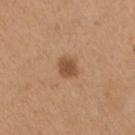The lesion was tiled from a total-body skin photograph and was not biopsied.
A lesion tile, about 15 mm wide, cut from a 3D total-body photograph.
The patient is a female aged 38 to 42.
The lesion's longest dimension is about 3 mm.
The lesion is located on the arm.
An algorithmic analysis of the crop reported an average lesion color of about L≈51 a*≈20 b*≈34 (CIELAB) and a lesion–skin lightness drop of about 11. It also reported border irregularity of about 2 on a 0–10 scale, a color-variation rating of about 2/10, and a peripheral color-asymmetry measure near 0.5.
This is a white-light tile.